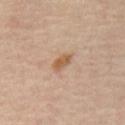Case summary:
- notes: imaged on a skin check; not biopsied
- image source: ~15 mm tile from a whole-body skin photo
- subject: in their mid- to late 50s
- automated lesion analysis: a lesion area of about 4 mm² and an eccentricity of roughly 0.8; a mean CIELAB color near L≈56 a*≈19 b*≈33, roughly 9 lightness units darker than nearby skin, and a normalized lesion–skin contrast near 8; a border-irregularity rating of about 2.5/10, internal color variation of about 1.5 on a 0–10 scale, and peripheral color asymmetry of about 0.5; a nevus-likeness score of about 40/100
- site: the mid back
- lesion diameter: ~3 mm (longest diameter)